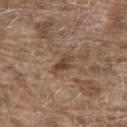Clinical impression:
Imaged during a routine full-body skin examination; the lesion was not biopsied and no histopathology is available.
Background:
Imaged with white-light lighting. A region of skin cropped from a whole-body photographic capture, roughly 15 mm wide. From the upper back. The recorded lesion diameter is about 3 mm. A male patient aged 78–82.